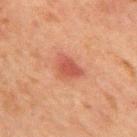notes=no biopsy performed (imaged during a skin exam); lesion diameter=~3.5 mm (longest diameter); anatomic site=the chest; subject=male, aged approximately 65; tile lighting=cross-polarized; image=total-body-photography crop, ~15 mm field of view.